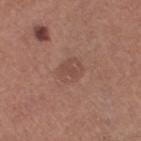| feature | finding |
|---|---|
| biopsy status | catalogued during a skin exam; not biopsied |
| TBP lesion metrics | border irregularity of about 1.5 on a 0–10 scale and internal color variation of about 1.5 on a 0–10 scale |
| tile lighting | white-light illumination |
| diameter | ≈3 mm |
| patient | female, about 50 years old |
| image source | ~15 mm tile from a whole-body skin photo |
| site | the right thigh |